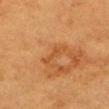No biopsy was performed on this lesion — it was imaged during a full skin examination and was not determined to be concerning. About 3.5 mm across. Cropped from a total-body skin-imaging series; the visible field is about 15 mm. The lesion is located on the head or neck. A male subject aged approximately 85 or older. This is a cross-polarized tile.